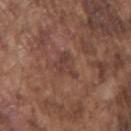<lesion>
  <biopsy_status>not biopsied; imaged during a skin examination</biopsy_status>
  <image>
    <source>total-body photography crop</source>
    <field_of_view_mm>15</field_of_view_mm>
  </image>
  <lighting>white-light</lighting>
  <patient>
    <sex>male</sex>
    <age_approx>75</age_approx>
  </patient>
  <site>arm</site>
  <lesion_size>
    <long_diameter_mm_approx>3.0</long_diameter_mm_approx>
  </lesion_size>
</lesion>